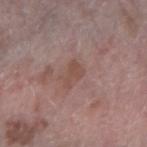Background: The lesion-visualizer software estimated a nevus-likeness score of about 0/100 and a lesion-detection confidence of about 100/100. A lesion tile, about 15 mm wide, cut from a 3D total-body photograph. The subject is a female aged 63 to 67. Approximately 3 mm at its widest. The lesion is on the right forearm.